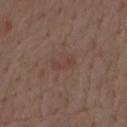Assessment: The lesion was tiled from a total-body skin photograph and was not biopsied. Acquisition and patient details: This is a white-light tile. The subject is a male aged 68–72. From the back. A 15 mm crop from a total-body photograph taken for skin-cancer surveillance. Longest diameter approximately 2.5 mm.